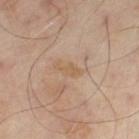The lesion's longest dimension is about 3 mm. Automated tile analysis of the lesion measured an area of roughly 3 mm², an eccentricity of roughly 0.9, and a shape-asymmetry score of about 0.4 (0 = symmetric). And it measured a lesion-detection confidence of about 100/100. From the left thigh. A 15 mm close-up extracted from a 3D total-body photography capture. Imaged with cross-polarized lighting. The subject is a male about 50 years old.